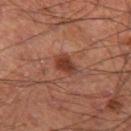notes: imaged on a skin check; not biopsied
subject: male, aged around 60
illumination: cross-polarized
imaging modality: 15 mm crop, total-body photography
location: the left thigh